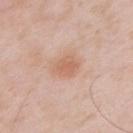Assessment: Captured during whole-body skin photography for melanoma surveillance; the lesion was not biopsied. Acquisition and patient details: The patient is a male aged approximately 55. Measured at roughly 3 mm in maximum diameter. The tile uses white-light illumination. Cropped from a total-body skin-imaging series; the visible field is about 15 mm. The lesion is on the chest.